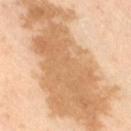| key | value |
|---|---|
| biopsy status | catalogued during a skin exam; not biopsied |
| diameter | about 18 mm |
| patient | male, aged 63 to 67 |
| image source | ~15 mm crop, total-body skin-cancer survey |
| body site | the right thigh |